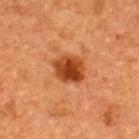The lesion was photographed on a routine skin check and not biopsied; there is no pathology result. This image is a 15 mm lesion crop taken from a total-body photograph. A male patient, roughly 50 years of age. The total-body-photography lesion software estimated a mean CIELAB color near L≈36 a*≈26 b*≈35, roughly 12 lightness units darker than nearby skin, and a normalized lesion–skin contrast near 10.5. And it measured a classifier nevus-likeness of about 95/100 and a lesion-detection confidence of about 100/100. This is a cross-polarized tile. On the back.